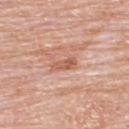Q: Was this lesion biopsied?
A: imaged on a skin check; not biopsied
Q: What is the lesion's diameter?
A: ≈3 mm
Q: Who is the patient?
A: male, aged approximately 80
Q: Lesion location?
A: the back
Q: How was this image acquired?
A: total-body-photography crop, ~15 mm field of view
Q: Automated lesion metrics?
A: an area of roughly 4.5 mm², an outline eccentricity of about 0.9 (0 = round, 1 = elongated), and a shape-asymmetry score of about 0.2 (0 = symmetric); a mean CIELAB color near L≈59 a*≈25 b*≈32, roughly 9 lightness units darker than nearby skin, and a normalized lesion–skin contrast near 6.5; a within-lesion color-variation index near 4/10 and peripheral color asymmetry of about 1.5
Q: Illumination type?
A: white-light